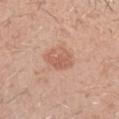Captured during whole-body skin photography for melanoma surveillance; the lesion was not biopsied.
About 3.5 mm across.
From the left forearm.
A male subject aged approximately 30.
Imaged with white-light lighting.
A lesion tile, about 15 mm wide, cut from a 3D total-body photograph.
Automated tile analysis of the lesion measured an area of roughly 7 mm², an eccentricity of roughly 0.6, and a shape-asymmetry score of about 0.3 (0 = symmetric). The analysis additionally found a lesion color around L≈59 a*≈23 b*≈30 in CIELAB, a lesion–skin lightness drop of about 9, and a lesion-to-skin contrast of about 6 (normalized; higher = more distinct). The software also gave a classifier nevus-likeness of about 20/100 and a detector confidence of about 100 out of 100 that the crop contains a lesion.